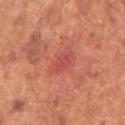No biopsy was performed on this lesion — it was imaged during a full skin examination and was not determined to be concerning. The subject is a male aged 63–67. Automated image analysis of the tile measured an eccentricity of roughly 0.75 and two-axis asymmetry of about 0.25. The analysis additionally found a mean CIELAB color near L≈52 a*≈36 b*≈31, roughly 7 lightness units darker than nearby skin, and a normalized border contrast of about 5. It also reported a border-irregularity rating of about 3/10, internal color variation of about 1 on a 0–10 scale, and radial color variation of about 0.5. The lesion is on the upper back. The tile uses white-light illumination. Cropped from a total-body skin-imaging series; the visible field is about 15 mm. The recorded lesion diameter is about 3 mm.